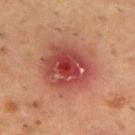follow-up: no biopsy performed (imaged during a skin exam)
tile lighting: cross-polarized
size: ≈6.5 mm
automated metrics: a footprint of about 29 mm², an outline eccentricity of about 0.4 (0 = round, 1 = elongated), and a symmetry-axis asymmetry near 0.15; a lesion color around L≈47 a*≈30 b*≈28 in CIELAB, about 11 CIELAB-L* units darker than the surrounding skin, and a lesion-to-skin contrast of about 8.5 (normalized; higher = more distinct); a border-irregularity index near 1.5/10, a color-variation rating of about 9.5/10, and peripheral color asymmetry of about 3; a classifier nevus-likeness of about 0/100 and lesion-presence confidence of about 100/100
patient: male, aged approximately 55
image: total-body-photography crop, ~15 mm field of view
site: the back